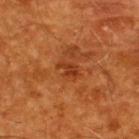notes: catalogued during a skin exam; not biopsied
lighting: cross-polarized
subject: male, aged 58–62
image source: ~15 mm crop, total-body skin-cancer survey
site: the back
diameter: ≈2.5 mm